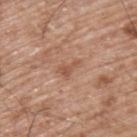Assessment:
No biopsy was performed on this lesion — it was imaged during a full skin examination and was not determined to be concerning.
Acquisition and patient details:
A male subject approximately 65 years of age. This image is a 15 mm lesion crop taken from a total-body photograph. The lesion is located on the upper back.